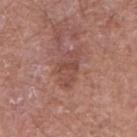Clinical impression: The lesion was tiled from a total-body skin photograph and was not biopsied. Image and clinical context: A male subject roughly 65 years of age. A roughly 15 mm field-of-view crop from a total-body skin photograph. The lesion-visualizer software estimated a lesion area of about 8 mm² and an eccentricity of roughly 0.85. The software also gave a mean CIELAB color near L≈48 a*≈23 b*≈25, about 8 CIELAB-L* units darker than the surrounding skin, and a lesion-to-skin contrast of about 6 (normalized; higher = more distinct). And it measured a border-irregularity rating of about 4/10, internal color variation of about 3 on a 0–10 scale, and peripheral color asymmetry of about 1. The analysis additionally found a classifier nevus-likeness of about 0/100. This is a white-light tile. Longest diameter approximately 4.5 mm. The lesion is on the right forearm.